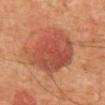The lesion was photographed on a routine skin check and not biopsied; there is no pathology result.
A roughly 15 mm field-of-view crop from a total-body skin photograph.
On the chest.
Longest diameter approximately 6.5 mm.
Automated image analysis of the tile measured a within-lesion color-variation index near 4.5/10 and peripheral color asymmetry of about 1.5. The software also gave a nevus-likeness score of about 100/100 and a detector confidence of about 100 out of 100 that the crop contains a lesion.
The patient is a male aged 58–62.